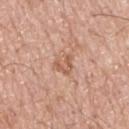Notes:
– notes: total-body-photography surveillance lesion; no biopsy
– patient: male, aged 68–72
– size: about 2.5 mm
– illumination: white-light illumination
– site: the upper back
– acquisition: ~15 mm crop, total-body skin-cancer survey